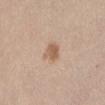Assessment: Imaged during a routine full-body skin examination; the lesion was not biopsied and no histopathology is available. Clinical summary: Automated image analysis of the tile measured a lesion area of about 5.5 mm² and two-axis asymmetry of about 0.25. And it measured a mean CIELAB color near L≈60 a*≈18 b*≈30 and a lesion-to-skin contrast of about 7 (normalized; higher = more distinct). The software also gave a classifier nevus-likeness of about 85/100 and a lesion-detection confidence of about 100/100. Located on the lower back. A female subject aged around 55. A roughly 15 mm field-of-view crop from a total-body skin photograph.